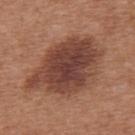  biopsy_status: not biopsied; imaged during a skin examination
  automated_metrics:
    shape_asymmetry: 0.2
    border_irregularity_0_10: 3.5
    color_variation_0_10: 4.5
    peripheral_color_asymmetry: 1.0
  lighting: white-light
  image:
    source: total-body photography crop
    field_of_view_mm: 15
  lesion_size:
    long_diameter_mm_approx: 9.5
  site: upper back
  patient:
    sex: female
    age_approx: 40